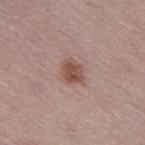Clinical impression: Captured during whole-body skin photography for melanoma surveillance; the lesion was not biopsied. Clinical summary: A female patient, in their 50s. Cropped from a whole-body photographic skin survey; the tile spans about 15 mm. Measured at roughly 3.5 mm in maximum diameter. This is a white-light tile. Automated tile analysis of the lesion measured a lesion area of about 6.5 mm², a shape eccentricity near 0.6, and a shape-asymmetry score of about 0.25 (0 = symmetric). The analysis additionally found an average lesion color of about L≈51 a*≈20 b*≈25 (CIELAB), roughly 10 lightness units darker than nearby skin, and a normalized lesion–skin contrast near 8. On the leg.